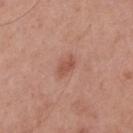| feature | finding |
|---|---|
| follow-up | imaged on a skin check; not biopsied |
| imaging modality | ~15 mm crop, total-body skin-cancer survey |
| body site | the mid back |
| illumination | white-light illumination |
| TBP lesion metrics | an area of roughly 4 mm² and an outline eccentricity of about 0.8 (0 = round, 1 = elongated); a border-irregularity rating of about 2.5/10 and a color-variation rating of about 1.5/10; a lesion-detection confidence of about 100/100 |
| size | about 3 mm |
| subject | male, aged around 55 |